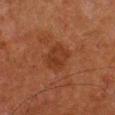The lesion was tiled from a total-body skin photograph and was not biopsied.
This image is a 15 mm lesion crop taken from a total-body photograph.
Approximately 3.5 mm at its widest.
The lesion is located on the right lower leg.
Automated image analysis of the tile measured border irregularity of about 1.5 on a 0–10 scale, a within-lesion color-variation index near 2/10, and radial color variation of about 1. And it measured a nevus-likeness score of about 10/100.
The subject is a male aged around 80.
The tile uses cross-polarized illumination.